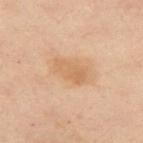Findings:
• body site · the chest
• lighting · cross-polarized illumination
• image · 15 mm crop, total-body photography
• patient · female, approximately 55 years of age